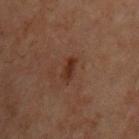Case summary:
– notes: imaged on a skin check; not biopsied
– automated metrics: a footprint of about 3 mm², an eccentricity of roughly 0.85, and two-axis asymmetry of about 0.25; an average lesion color of about L≈22 a*≈17 b*≈22 (CIELAB), roughly 7 lightness units darker than nearby skin, and a normalized lesion–skin contrast near 8
– body site: the chest
– acquisition: ~15 mm tile from a whole-body skin photo
– tile lighting: cross-polarized
– patient: male, aged 68–72
– lesion size: ≈2.5 mm